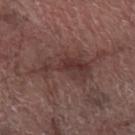Imaged during a routine full-body skin examination; the lesion was not biopsied and no histopathology is available.
Automated image analysis of the tile measured a lesion area of about 15 mm². The analysis additionally found an average lesion color of about L≈35 a*≈19 b*≈19 (CIELAB) and a normalized lesion–skin contrast near 7.
The lesion is located on the arm.
Cropped from a total-body skin-imaging series; the visible field is about 15 mm.
A male subject in their 70s.
About 7.5 mm across.
The tile uses white-light illumination.